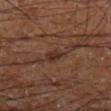Q: Lesion location?
A: the left lower leg
Q: Illumination type?
A: cross-polarized illumination
Q: What is the lesion's diameter?
A: about 2.5 mm
Q: What kind of image is this?
A: total-body-photography crop, ~15 mm field of view
Q: Patient demographics?
A: male, about 60 years old
Q: What did automated image analysis measure?
A: a lesion area of about 4.5 mm²; a lesion–skin lightness drop of about 7 and a normalized border contrast of about 7